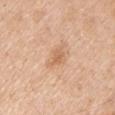The lesion was photographed on a routine skin check and not biopsied; there is no pathology result.